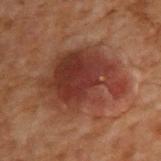Findings:
• notes — total-body-photography surveillance lesion; no biopsy
• automated metrics — an average lesion color of about L≈27 a*≈20 b*≈22 (CIELAB), roughly 9 lightness units darker than nearby skin, and a normalized border contrast of about 9
• site — the upper back
• image source — ~15 mm tile from a whole-body skin photo
• tile lighting — cross-polarized
• diameter — ≈8.5 mm
• patient — male, about 70 years old